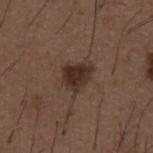{"biopsy_status": "not biopsied; imaged during a skin examination", "lighting": "white-light", "image": {"source": "total-body photography crop", "field_of_view_mm": 15}, "lesion_size": {"long_diameter_mm_approx": 4.0}, "patient": {"sex": "male", "age_approx": 50}, "site": "front of the torso"}